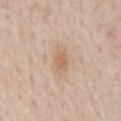follow-up=no biopsy performed (imaged during a skin exam) | tile lighting=white-light | lesion diameter=about 3.5 mm | location=the chest | image-analysis metrics=a lesion area of about 5.5 mm² and a shape eccentricity near 0.8; a mean CIELAB color near L≈64 a*≈17 b*≈32, a lesion–skin lightness drop of about 8, and a normalized lesion–skin contrast near 6; a lesion-detection confidence of about 100/100 | acquisition=15 mm crop, total-body photography | subject=male, aged around 75.